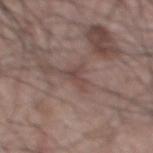A male patient, in their mid-60s. The lesion's longest dimension is about 3 mm. A close-up tile cropped from a whole-body skin photograph, about 15 mm across. Captured under white-light illumination. The lesion-visualizer software estimated an area of roughly 4 mm², a shape eccentricity near 0.45, and a shape-asymmetry score of about 0.6 (0 = symmetric). The lesion is located on the front of the torso.